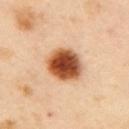follow-up = total-body-photography surveillance lesion; no biopsy | anatomic site = the left arm | patient = female, about 40 years old | imaging modality = total-body-photography crop, ~15 mm field of view.